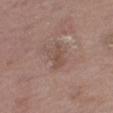Located on the right thigh. A region of skin cropped from a whole-body photographic capture, roughly 15 mm wide. A male patient aged 73 to 77.